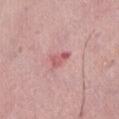No biopsy was performed on this lesion — it was imaged during a full skin examination and was not determined to be concerning. The subject is a female aged around 65. A 15 mm close-up extracted from a 3D total-body photography capture. The lesion is located on the front of the torso. The lesion-visualizer software estimated a shape eccentricity near 0.85 and two-axis asymmetry of about 0.55. The software also gave an average lesion color of about L≈58 a*≈30 b*≈21 (CIELAB) and a lesion-to-skin contrast of about 7 (normalized; higher = more distinct). It also reported border irregularity of about 5 on a 0–10 scale, a color-variation rating of about 0.5/10, and radial color variation of about 0.